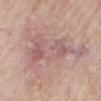Q: Was a biopsy performed?
A: no biopsy performed (imaged during a skin exam)
Q: How large is the lesion?
A: about 9 mm
Q: Who is the patient?
A: male, aged 63 to 67
Q: Where on the body is the lesion?
A: the chest
Q: What is the imaging modality?
A: ~15 mm crop, total-body skin-cancer survey